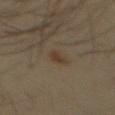Case summary:
- follow-up: catalogued during a skin exam; not biopsied
- lighting: cross-polarized illumination
- image source: ~15 mm tile from a whole-body skin photo
- body site: the mid back
- size: ≈3 mm
- patient: male, aged 33–37